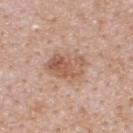workup = catalogued during a skin exam; not biopsied | patient = male, aged approximately 40 | image = ~15 mm crop, total-body skin-cancer survey | body site = the upper back | image-analysis metrics = a footprint of about 13 mm², an eccentricity of roughly 0.65, and a symmetry-axis asymmetry near 0.2; a border-irregularity index near 3/10, internal color variation of about 6 on a 0–10 scale, and a peripheral color-asymmetry measure near 2.5 | lighting = white-light illumination.